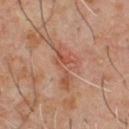Impression: Recorded during total-body skin imaging; not selected for excision or biopsy. Background: From the chest. Imaged with cross-polarized lighting. Measured at roughly 6.5 mm in maximum diameter. A roughly 15 mm field-of-view crop from a total-body skin photograph. A male subject aged 58 to 62.